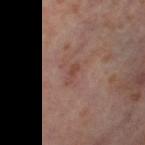| feature | finding |
|---|---|
| follow-up | no biopsy performed (imaged during a skin exam) |
| site | the right thigh |
| diameter | ≈2.5 mm |
| image source | total-body-photography crop, ~15 mm field of view |
| illumination | cross-polarized illumination |
| patient | female, aged 53–57 |